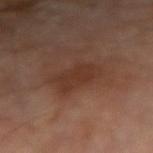This lesion was catalogued during total-body skin photography and was not selected for biopsy. A male subject, approximately 70 years of age. The tile uses cross-polarized illumination. Automated tile analysis of the lesion measured an average lesion color of about L≈33 a*≈20 b*≈26 (CIELAB), roughly 7 lightness units darker than nearby skin, and a normalized border contrast of about 6.5. The software also gave a border-irregularity index near 3.5/10, a within-lesion color-variation index near 2/10, and radial color variation of about 0.5. The analysis additionally found a classifier nevus-likeness of about 10/100 and a detector confidence of about 100 out of 100 that the crop contains a lesion. Approximately 5 mm at its widest. The lesion is on the arm. A lesion tile, about 15 mm wide, cut from a 3D total-body photograph.